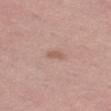<tbp_lesion>
  <image>
    <source>total-body photography crop</source>
    <field_of_view_mm>15</field_of_view_mm>
  </image>
  <patient>
    <sex>female</sex>
    <age_approx>45</age_approx>
  </patient>
  <lesion_size>
    <long_diameter_mm_approx>2.5</long_diameter_mm_approx>
  </lesion_size>
  <lighting>white-light</lighting>
  <site>left thigh</site>
</tbp_lesion>